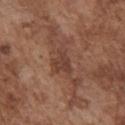| key | value |
|---|---|
| notes | catalogued during a skin exam; not biopsied |
| patient | male, in their mid- to late 70s |
| acquisition | 15 mm crop, total-body photography |
| lesion diameter | about 3.5 mm |
| site | the chest |
| image-analysis metrics | a mean CIELAB color near L≈41 a*≈21 b*≈26, roughly 8 lightness units darker than nearby skin, and a normalized lesion–skin contrast near 6.5 |